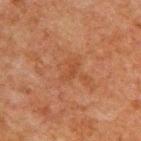A male patient aged 58–62. Measured at roughly 2.5 mm in maximum diameter. On the upper back. A lesion tile, about 15 mm wide, cut from a 3D total-body photograph.